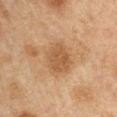notes: imaged on a skin check; not biopsied
lighting: cross-polarized illumination
automated metrics: a lesion color around L≈42 a*≈16 b*≈30 in CIELAB, about 7 CIELAB-L* units darker than the surrounding skin, and a lesion-to-skin contrast of about 6.5 (normalized; higher = more distinct); a border-irregularity rating of about 2/10, a color-variation rating of about 2/10, and a peripheral color-asymmetry measure near 0.5
subject: male, in their 50s
body site: the left upper arm
acquisition: total-body-photography crop, ~15 mm field of view